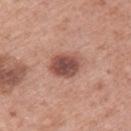Recorded during total-body skin imaging; not selected for excision or biopsy. The lesion's longest dimension is about 4 mm. The lesion-visualizer software estimated a lesion color around L≈50 a*≈23 b*≈26 in CIELAB, about 15 CIELAB-L* units darker than the surrounding skin, and a normalized border contrast of about 10. The analysis additionally found a border-irregularity index near 1.5/10 and peripheral color asymmetry of about 1. It also reported a detector confidence of about 100 out of 100 that the crop contains a lesion. A female subject, about 50 years old. The lesion is on the left upper arm. The tile uses white-light illumination. This image is a 15 mm lesion crop taken from a total-body photograph.